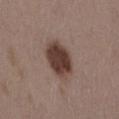The recorded lesion diameter is about 4.5 mm. A 15 mm close-up tile from a total-body photography series done for melanoma screening. Located on the mid back. The subject is a female in their mid- to late 30s.